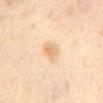{"biopsy_status": "not biopsied; imaged during a skin examination", "automated_metrics": {"shape_asymmetry": 0.25, "border_irregularity_0_10": 2.5, "color_variation_0_10": 2.0, "nevus_likeness_0_100": 60, "lesion_detection_confidence_0_100": 100}, "image": {"source": "total-body photography crop", "field_of_view_mm": 15}, "site": "mid back", "lesion_size": {"long_diameter_mm_approx": 3.0}, "lighting": "cross-polarized", "patient": {"sex": "female", "age_approx": 55}}